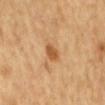size=~2.5 mm (longest diameter) | anatomic site=the mid back | patient=male, in their mid- to late 60s | automated metrics=a lesion–skin lightness drop of about 11 and a normalized border contrast of about 8; a peripheral color-asymmetry measure near 0.5; a nevus-likeness score of about 45/100 and lesion-presence confidence of about 100/100 | acquisition=15 mm crop, total-body photography | lighting=cross-polarized illumination.